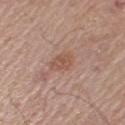Q: Was a biopsy performed?
A: catalogued during a skin exam; not biopsied